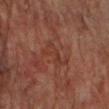Imaged during a routine full-body skin examination; the lesion was not biopsied and no histopathology is available.
A male patient, in their mid-70s.
The lesion is located on the right upper arm.
Cropped from a whole-body photographic skin survey; the tile spans about 15 mm.
This is a cross-polarized tile.